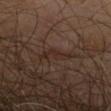notes: no biopsy performed (imaged during a skin exam) | lesion diameter: about 4 mm | lighting: cross-polarized illumination | subject: male, aged around 65 | imaging modality: ~15 mm crop, total-body skin-cancer survey | body site: the right upper arm.